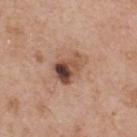image source: 15 mm crop, total-body photography | image-analysis metrics: a lesion area of about 9.5 mm², an outline eccentricity of about 0.65 (0 = round, 1 = elongated), and a shape-asymmetry score of about 0.3 (0 = symmetric); border irregularity of about 3 on a 0–10 scale, internal color variation of about 10 on a 0–10 scale, and a peripheral color-asymmetry measure near 6; a classifier nevus-likeness of about 5/100 and a lesion-detection confidence of about 100/100 | illumination: white-light illumination | subject: male, roughly 55 years of age | size: ~4 mm (longest diameter) | body site: the upper back.